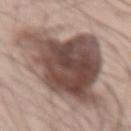Recorded during total-body skin imaging; not selected for excision or biopsy.
The lesion's longest dimension is about 11.5 mm.
Cropped from a whole-body photographic skin survey; the tile spans about 15 mm.
A male subject, aged 53 to 57.
Located on the abdomen.